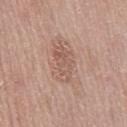Q: Is there a histopathology result?
A: catalogued during a skin exam; not biopsied
Q: What lighting was used for the tile?
A: white-light
Q: Lesion location?
A: the mid back
Q: How large is the lesion?
A: ~4.5 mm (longest diameter)
Q: Who is the patient?
A: female, about 60 years old
Q: How was this image acquired?
A: total-body-photography crop, ~15 mm field of view